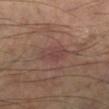Clinical impression: This lesion was catalogued during total-body skin photography and was not selected for biopsy. Background: About 4 mm across. The subject is a male about 60 years old. The lesion is located on the left lower leg. A region of skin cropped from a whole-body photographic capture, roughly 15 mm wide.